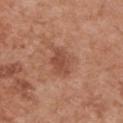biopsy status = catalogued during a skin exam; not biopsied.